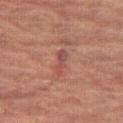The lesion was tiled from a total-body skin photograph and was not biopsied. Imaged with cross-polarized lighting. Automated tile analysis of the lesion measured an area of roughly 3.5 mm², an eccentricity of roughly 0.95, and two-axis asymmetry of about 0.3. The analysis additionally found an average lesion color of about L≈40 a*≈23 b*≈21 (CIELAB), roughly 6 lightness units darker than nearby skin, and a normalized lesion–skin contrast near 6. And it measured a border-irregularity index near 3.5/10, a within-lesion color-variation index near 1/10, and a peripheral color-asymmetry measure near 0.5. Measured at roughly 3.5 mm in maximum diameter. A 15 mm crop from a total-body photograph taken for skin-cancer surveillance. The lesion is on the right thigh. A female subject aged 68–72.